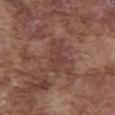Clinical summary:
Cropped from a total-body skin-imaging series; the visible field is about 15 mm. From the abdomen. The recorded lesion diameter is about 4 mm. An algorithmic analysis of the crop reported about 6 CIELAB-L* units darker than the surrounding skin and a normalized border contrast of about 5. Captured under white-light illumination. The subject is a male aged 73 to 77.